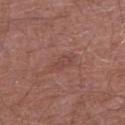Impression: Recorded during total-body skin imaging; not selected for excision or biopsy. Acquisition and patient details: The tile uses white-light illumination. The patient is a male roughly 70 years of age. A 15 mm crop from a total-body photograph taken for skin-cancer surveillance. Approximately 3 mm at its widest. From the left lower leg.